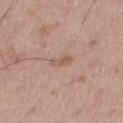Case summary:
- follow-up — catalogued during a skin exam; not biopsied
- acquisition — total-body-photography crop, ~15 mm field of view
- lesion diameter — about 2.5 mm
- subject — male, aged around 55
- anatomic site — the right thigh